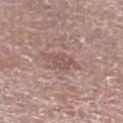The lesion was photographed on a routine skin check and not biopsied; there is no pathology result. About 3 mm across. The tile uses white-light illumination. Located on the leg. A male subject in their 80s. A roughly 15 mm field-of-view crop from a total-body skin photograph. An algorithmic analysis of the crop reported a lesion area of about 4.5 mm², an eccentricity of roughly 0.7, and two-axis asymmetry of about 0.35. The software also gave a mean CIELAB color near L≈52 a*≈18 b*≈23, roughly 8 lightness units darker than nearby skin, and a normalized lesion–skin contrast near 6. The software also gave a border-irregularity rating of about 3.5/10, a within-lesion color-variation index near 1.5/10, and radial color variation of about 0.5.